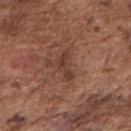Clinical impression: No biopsy was performed on this lesion — it was imaged during a full skin examination and was not determined to be concerning. Background: An algorithmic analysis of the crop reported a lesion color around L≈38 a*≈21 b*≈23 in CIELAB, roughly 9 lightness units darker than nearby skin, and a normalized lesion–skin contrast near 8. Approximately 3.5 mm at its widest. A 15 mm crop from a total-body photograph taken for skin-cancer surveillance. A male subject about 75 years old. This is a white-light tile. From the arm.